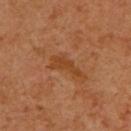The lesion was photographed on a routine skin check and not biopsied; there is no pathology result. Captured under cross-polarized illumination. Longest diameter approximately 4 mm. A male patient approximately 60 years of age. The lesion is on the upper back. A 15 mm close-up tile from a total-body photography series done for melanoma screening.